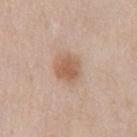This lesion was catalogued during total-body skin photography and was not selected for biopsy.
Cropped from a whole-body photographic skin survey; the tile spans about 15 mm.
Approximately 3.5 mm at its widest.
From the front of the torso.
A male subject, in their 60s.
Captured under white-light illumination.
Automated tile analysis of the lesion measured a footprint of about 8.5 mm² and an eccentricity of roughly 0.55. It also reported an automated nevus-likeness rating near 80 out of 100.